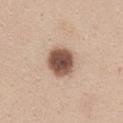No biopsy was performed on this lesion — it was imaged during a full skin examination and was not determined to be concerning. The lesion is on the chest. The patient is a female aged 38 to 42. Automated tile analysis of the lesion measured an average lesion color of about L≈52 a*≈19 b*≈27 (CIELAB), a lesion–skin lightness drop of about 19, and a lesion-to-skin contrast of about 12.5 (normalized; higher = more distinct). The analysis additionally found an automated nevus-likeness rating near 90 out of 100 and a detector confidence of about 100 out of 100 that the crop contains a lesion. Captured under white-light illumination. A 15 mm close-up tile from a total-body photography series done for melanoma screening. Approximately 4 mm at its widest.